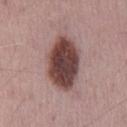notes = total-body-photography surveillance lesion; no biopsy.